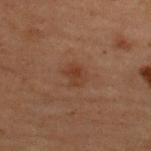The lesion was photographed on a routine skin check and not biopsied; there is no pathology result. An algorithmic analysis of the crop reported a lesion area of about 5 mm², a shape eccentricity near 0.55, and two-axis asymmetry of about 0.2. The software also gave a lesion color around L≈29 a*≈17 b*≈24 in CIELAB and a lesion-to-skin contrast of about 5.5 (normalized; higher = more distinct). It also reported a border-irregularity rating of about 2/10. And it measured a classifier nevus-likeness of about 35/100 and a lesion-detection confidence of about 100/100. A 15 mm close-up extracted from a 3D total-body photography capture. A male patient aged approximately 60. The lesion's longest dimension is about 3 mm. Located on the upper back.